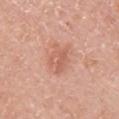<tbp_lesion>
<lesion_size>
  <long_diameter_mm_approx>4.0</long_diameter_mm_approx>
</lesion_size>
<site>upper back</site>
<image>
  <source>total-body photography crop</source>
  <field_of_view_mm>15</field_of_view_mm>
</image>
<patient>
  <sex>male</sex>
  <age_approx>60</age_approx>
</patient>
</tbp_lesion>